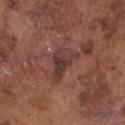| key | value |
|---|---|
| biopsy status | no biopsy performed (imaged during a skin exam) |
| image | ~15 mm crop, total-body skin-cancer survey |
| tile lighting | white-light illumination |
| location | the chest |
| subject | male, aged 73–77 |